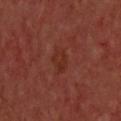Clinical impression:
Recorded during total-body skin imaging; not selected for excision or biopsy.
Acquisition and patient details:
A male subject roughly 65 years of age. Located on the upper back. Captured under cross-polarized illumination. The recorded lesion diameter is about 3 mm. The total-body-photography lesion software estimated an eccentricity of roughly 0.75 and a symmetry-axis asymmetry near 0.2. The software also gave a lesion–skin lightness drop of about 4. And it measured border irregularity of about 2 on a 0–10 scale and radial color variation of about 1. The analysis additionally found an automated nevus-likeness rating near 0 out of 100 and a lesion-detection confidence of about 100/100. A lesion tile, about 15 mm wide, cut from a 3D total-body photograph.